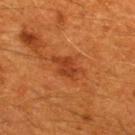| feature | finding |
|---|---|
| workup | catalogued during a skin exam; not biopsied |
| image source | ~15 mm crop, total-body skin-cancer survey |
| site | the upper back |
| automated metrics | an average lesion color of about L≈39 a*≈29 b*≈38 (CIELAB) and roughly 8 lightness units darker than nearby skin; lesion-presence confidence of about 100/100 |
| tile lighting | cross-polarized illumination |
| lesion diameter | ~4 mm (longest diameter) |
| patient | male, aged approximately 60 |